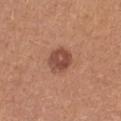Clinical impression:
Imaged during a routine full-body skin examination; the lesion was not biopsied and no histopathology is available.
Acquisition and patient details:
The lesion is on the arm. A 15 mm close-up tile from a total-body photography series done for melanoma screening. A female subject, aged approximately 25.